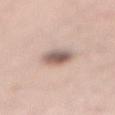The patient is a male aged approximately 60.
On the abdomen.
The recorded lesion diameter is about 3.5 mm.
The tile uses white-light illumination.
A region of skin cropped from a whole-body photographic capture, roughly 15 mm wide.
An algorithmic analysis of the crop reported a footprint of about 7.5 mm², an outline eccentricity of about 0.65 (0 = round, 1 = elongated), and a symmetry-axis asymmetry near 0.1. And it measured a border-irregularity index near 1.5/10, a within-lesion color-variation index near 6.5/10, and a peripheral color-asymmetry measure near 2. The software also gave lesion-presence confidence of about 100/100.